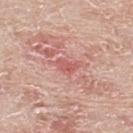The lesion was tiled from a total-body skin photograph and was not biopsied.
This image is a 15 mm lesion crop taken from a total-body photograph.
Located on the back.
The subject is a male aged 63 to 67.
The tile uses white-light illumination.
Measured at roughly 2.5 mm in maximum diameter.
The total-body-photography lesion software estimated an area of roughly 3.5 mm² and an eccentricity of roughly 0.8. The software also gave a classifier nevus-likeness of about 0/100 and a lesion-detection confidence of about 100/100.